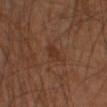No biopsy was performed on this lesion — it was imaged during a full skin examination and was not determined to be concerning.
A male subject, aged approximately 45.
A region of skin cropped from a whole-body photographic capture, roughly 15 mm wide.
On the left forearm.